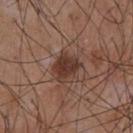{
  "biopsy_status": "not biopsied; imaged during a skin examination",
  "site": "abdomen",
  "patient": {
    "sex": "male",
    "age_approx": 55
  },
  "image": {
    "source": "total-body photography crop",
    "field_of_view_mm": 15
  },
  "lighting": "white-light",
  "automated_metrics": {
    "border_irregularity_0_10": 2.5,
    "color_variation_0_10": 3.0,
    "peripheral_color_asymmetry": 1.0
  },
  "lesion_size": {
    "long_diameter_mm_approx": 3.5
  }
}